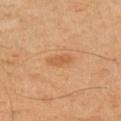notes = catalogued during a skin exam; not biopsied
TBP lesion metrics = a footprint of about 4 mm², a shape eccentricity near 0.8, and two-axis asymmetry of about 0.25; lesion-presence confidence of about 100/100
patient = male, aged 38 to 42
illumination = cross-polarized
lesion size = ≈2.5 mm
image = ~15 mm crop, total-body skin-cancer survey
anatomic site = the arm